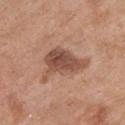Notes:
- lesion diameter: about 6 mm
- acquisition: ~15 mm crop, total-body skin-cancer survey
- location: the chest
- subject: female, roughly 65 years of age
- tile lighting: white-light